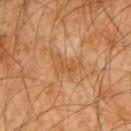No biopsy was performed on this lesion — it was imaged during a full skin examination and was not determined to be concerning. A roughly 15 mm field-of-view crop from a total-body skin photograph. The lesion is located on the right upper arm. A male patient in their 60s. The tile uses cross-polarized illumination.